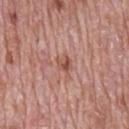Clinical impression:
No biopsy was performed on this lesion — it was imaged during a full skin examination and was not determined to be concerning.
Clinical summary:
Located on the mid back. Cropped from a total-body skin-imaging series; the visible field is about 15 mm. Captured under white-light illumination. Automated image analysis of the tile measured a lesion area of about 3.5 mm², an outline eccentricity of about 0.7 (0 = round, 1 = elongated), and a symmetry-axis asymmetry near 0.35. The software also gave internal color variation of about 4.5 on a 0–10 scale and radial color variation of about 2. The patient is a male aged 68 to 72.